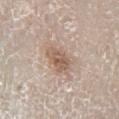| feature | finding |
|---|---|
| workup | total-body-photography surveillance lesion; no biopsy |
| subject | male, in their 60s |
| imaging modality | 15 mm crop, total-body photography |
| image-analysis metrics | a border-irregularity rating of about 2/10, a color-variation rating of about 4/10, and a peripheral color-asymmetry measure near 1.5; a classifier nevus-likeness of about 95/100 and a lesion-detection confidence of about 100/100 |
| illumination | white-light illumination |
| site | the right lower leg |
| diameter | about 4 mm |